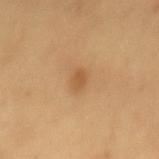Recorded during total-body skin imaging; not selected for excision or biopsy. The lesion-visualizer software estimated a lesion–skin lightness drop of about 8 and a normalized border contrast of about 6.5. And it measured a border-irregularity rating of about 2.5/10, a within-lesion color-variation index near 1/10, and radial color variation of about 0.5. From the mid back. A close-up tile cropped from a whole-body skin photograph, about 15 mm across. Imaged with cross-polarized lighting. Measured at roughly 2 mm in maximum diameter. The patient is a female in their 40s.